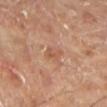<case>
<biopsy_status>not biopsied; imaged during a skin examination</biopsy_status>
<patient>
  <sex>male</sex>
  <age_approx>70</age_approx>
</patient>
<image>
  <source>total-body photography crop</source>
  <field_of_view_mm>15</field_of_view_mm>
</image>
<site>left lower leg</site>
</case>